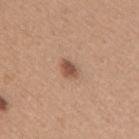notes = total-body-photography surveillance lesion; no biopsy
site = the back
diameter = ≈2.5 mm
image = ~15 mm crop, total-body skin-cancer survey
subject = female, aged 28–32
tile lighting = white-light illumination